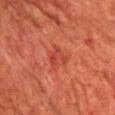Findings:
- image — total-body-photography crop, ~15 mm field of view
- body site — the chest
- subject — male, in their 70s
- automated lesion analysis — an area of roughly 5 mm², an outline eccentricity of about 0.6 (0 = round, 1 = elongated), and two-axis asymmetry of about 0.25
- lesion size — ≈3 mm